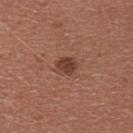biopsy_status: not biopsied; imaged during a skin examination
image:
  source: total-body photography crop
  field_of_view_mm: 15
lesion_size:
  long_diameter_mm_approx: 2.5
patient:
  sex: female
  age_approx: 25
automated_metrics:
  cielab_L: 41
  cielab_a: 23
  cielab_b: 27
lighting: white-light
site: upper back